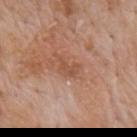diameter: about 2.5 mm
automated lesion analysis: an area of roughly 3 mm², an outline eccentricity of about 0.8 (0 = round, 1 = elongated), and a symmetry-axis asymmetry near 0.5; about 7 CIELAB-L* units darker than the surrounding skin and a normalized lesion–skin contrast near 5.5; a border-irregularity index near 5.5/10 and internal color variation of about 0 on a 0–10 scale; an automated nevus-likeness rating near 0 out of 100 and a detector confidence of about 100 out of 100 that the crop contains a lesion
subject: male, aged approximately 60
tile lighting: white-light illumination
body site: the mid back
imaging modality: total-body-photography crop, ~15 mm field of view